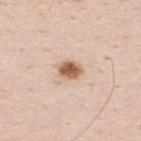{
  "biopsy_status": "not biopsied; imaged during a skin examination",
  "patient": {
    "sex": "male",
    "age_approx": 35
  },
  "site": "upper back",
  "lesion_size": {
    "long_diameter_mm_approx": 2.5
  },
  "image": {
    "source": "total-body photography crop",
    "field_of_view_mm": 15
  },
  "lighting": "white-light",
  "automated_metrics": {
    "border_irregularity_0_10": 1.5,
    "color_variation_0_10": 3.5,
    "peripheral_color_asymmetry": 1.5
  }
}